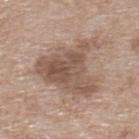workup: imaged on a skin check; not biopsied
patient: female, approximately 75 years of age
image: 15 mm crop, total-body photography
tile lighting: white-light
automated metrics: an area of roughly 29 mm² and a symmetry-axis asymmetry near 0.5; an average lesion color of about L≈53 a*≈16 b*≈26 (CIELAB), a lesion–skin lightness drop of about 11, and a normalized lesion–skin contrast near 7.5
diameter: ~8 mm (longest diameter)
body site: the back